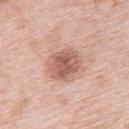{
  "biopsy_status": "not biopsied; imaged during a skin examination",
  "patient": {
    "sex": "female",
    "age_approx": 65
  },
  "lighting": "white-light",
  "image": {
    "source": "total-body photography crop",
    "field_of_view_mm": 15
  },
  "site": "upper back"
}